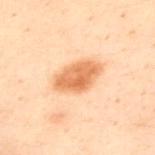{"biopsy_status": "not biopsied; imaged during a skin examination", "image": {"source": "total-body photography crop", "field_of_view_mm": 15}, "patient": {"sex": "male", "age_approx": 30}, "lighting": "cross-polarized", "site": "upper back"}